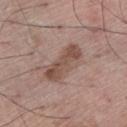This lesion was catalogued during total-body skin photography and was not selected for biopsy.
A 15 mm crop from a total-body photograph taken for skin-cancer surveillance.
The lesion is located on the left lower leg.
Imaged with white-light lighting.
Longest diameter approximately 5.5 mm.
A male patient, roughly 70 years of age.